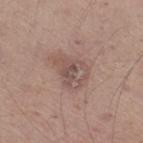Acquisition and patient details:
The lesion is on the right thigh. The patient is a male approximately 60 years of age. The lesion's longest dimension is about 4.5 mm. Imaged with white-light lighting. A close-up tile cropped from a whole-body skin photograph, about 15 mm across.